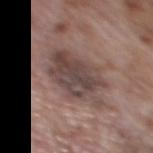No biopsy was performed on this lesion — it was imaged during a full skin examination and was not determined to be concerning.
Captured under white-light illumination.
About 6 mm across.
On the mid back.
A 15 mm crop from a total-body photograph taken for skin-cancer surveillance.
A male patient aged around 70.